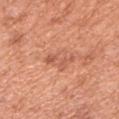<lesion>
  <biopsy_status>not biopsied; imaged during a skin examination</biopsy_status>
  <site>arm</site>
  <patient>
    <sex>male</sex>
    <age_approx>65</age_approx>
  </patient>
  <image>
    <source>total-body photography crop</source>
    <field_of_view_mm>15</field_of_view_mm>
  </image>
</lesion>